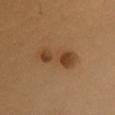Clinical impression: Imaged during a routine full-body skin examination; the lesion was not biopsied and no histopathology is available. Acquisition and patient details: A female patient in their mid- to late 30s. The lesion is located on the chest. A 15 mm crop from a total-body photograph taken for skin-cancer surveillance.